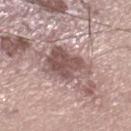Notes:
– biopsy status — total-body-photography surveillance lesion; no biopsy